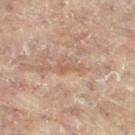Part of a total-body skin-imaging series; this lesion was reviewed on a skin check and was not flagged for biopsy. Captured under cross-polarized illumination. Located on the right leg. A 15 mm close-up tile from a total-body photography series done for melanoma screening. The patient is a female aged around 80. Approximately 3 mm at its widest.